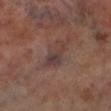• follow-up · catalogued during a skin exam; not biopsied
• subject · male, approximately 70 years of age
• location · the left lower leg
• acquisition · ~15 mm tile from a whole-body skin photo